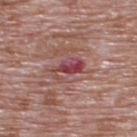No biopsy was performed on this lesion — it was imaged during a full skin examination and was not determined to be concerning. Cropped from a total-body skin-imaging series; the visible field is about 15 mm. The subject is a male aged 68 to 72. Located on the upper back. This is a white-light tile.